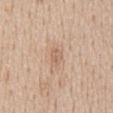A male subject aged 58 to 62. From the chest. Longest diameter approximately 2.5 mm. This image is a 15 mm lesion crop taken from a total-body photograph. Imaged with white-light lighting.